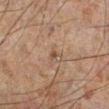The lesion was photographed on a routine skin check and not biopsied; there is no pathology result. The lesion is on the left leg. This is a cross-polarized tile. An algorithmic analysis of the crop reported a lesion area of about 1 mm², an eccentricity of roughly 0.6, and two-axis asymmetry of about 0.5. It also reported an average lesion color of about L≈35 a*≈15 b*≈24 (CIELAB), roughly 7 lightness units darker than nearby skin, and a normalized lesion–skin contrast near 7.5. And it measured a lesion-detection confidence of about 100/100. The recorded lesion diameter is about 1 mm. Cropped from a whole-body photographic skin survey; the tile spans about 15 mm. The subject is a male aged around 60.